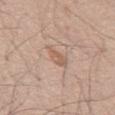Assessment: Recorded during total-body skin imaging; not selected for excision or biopsy. Clinical summary: An algorithmic analysis of the crop reported an eccentricity of roughly 0.8 and a shape-asymmetry score of about 0.3 (0 = symmetric). It also reported a nevus-likeness score of about 0/100 and a detector confidence of about 100 out of 100 that the crop contains a lesion. On the front of the torso. A male patient aged around 60. A close-up tile cropped from a whole-body skin photograph, about 15 mm across. About 3 mm across. This is a white-light tile.